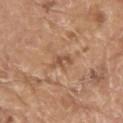workup=total-body-photography surveillance lesion; no biopsy
size=≈3 mm
imaging modality=~15 mm tile from a whole-body skin photo
body site=the left upper arm
patient=male, roughly 80 years of age
lighting=white-light